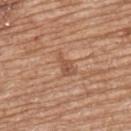notes: imaged on a skin check; not biopsied
patient: female, approximately 70 years of age
imaging modality: ~15 mm crop, total-body skin-cancer survey
TBP lesion metrics: an average lesion color of about L≈54 a*≈22 b*≈32 (CIELAB), roughly 7 lightness units darker than nearby skin, and a lesion-to-skin contrast of about 5.5 (normalized; higher = more distinct); border irregularity of about 5 on a 0–10 scale and a color-variation rating of about 2.5/10; an automated nevus-likeness rating near 0 out of 100
body site: the upper back
tile lighting: white-light
size: about 3.5 mm